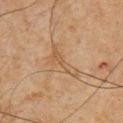This lesion was catalogued during total-body skin photography and was not selected for biopsy.
The subject is a male aged 63–67.
The lesion is located on the chest.
A lesion tile, about 15 mm wide, cut from a 3D total-body photograph.